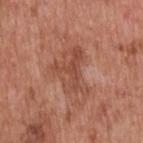follow-up — imaged on a skin check; not biopsied
lesion diameter — ≈6 mm
image — ~15 mm crop, total-body skin-cancer survey
site — the back
subject — male, roughly 60 years of age
image-analysis metrics — a border-irregularity index near 6/10, a within-lesion color-variation index near 4/10, and radial color variation of about 1.5; a classifier nevus-likeness of about 0/100 and lesion-presence confidence of about 100/100
tile lighting — white-light